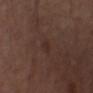workup — no biopsy performed (imaged during a skin exam)
patient — male, roughly 75 years of age
image — ~15 mm tile from a whole-body skin photo
diameter — ~2.5 mm (longest diameter)
location — the chest
image-analysis metrics — border irregularity of about 3 on a 0–10 scale, a within-lesion color-variation index near 0.5/10, and a peripheral color-asymmetry measure near 0; a nevus-likeness score of about 0/100